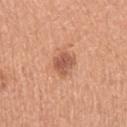Clinical impression: Imaged during a routine full-body skin examination; the lesion was not biopsied and no histopathology is available. Background: From the left upper arm. A female patient roughly 65 years of age. A region of skin cropped from a whole-body photographic capture, roughly 15 mm wide.